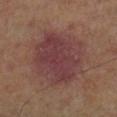  biopsy_status: not biopsied; imaged during a skin examination
  image:
    source: total-body photography crop
    field_of_view_mm: 15
  patient:
    sex: male
    age_approx: 65
  lighting: cross-polarized
  automated_metrics:
    cielab_L: 34
    cielab_a: 20
    cielab_b: 17
    vs_skin_darker_L: 7.0
    vs_skin_contrast_norm: 8.5
    nevus_likeness_0_100: 0
    lesion_detection_confidence_0_100: 100
  site: right lower leg